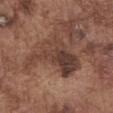Captured during whole-body skin photography for melanoma surveillance; the lesion was not biopsied.
Automated image analysis of the tile measured an area of roughly 20 mm², an eccentricity of roughly 0.7, and two-axis asymmetry of about 0.45. And it measured a mean CIELAB color near L≈40 a*≈19 b*≈24, roughly 10 lightness units darker than nearby skin, and a normalized border contrast of about 8.
This image is a 15 mm lesion crop taken from a total-body photograph.
The lesion is located on the front of the torso.
The patient is a male aged 73 to 77.
Imaged with white-light lighting.
Approximately 7 mm at its widest.